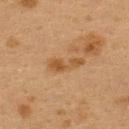Captured during whole-body skin photography for melanoma surveillance; the lesion was not biopsied.
On the upper back.
Longest diameter approximately 4.5 mm.
A female patient, aged approximately 40.
A close-up tile cropped from a whole-body skin photograph, about 15 mm across.